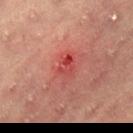Q: Lesion location?
A: the lower back
Q: Illumination type?
A: cross-polarized
Q: How was this image acquired?
A: ~15 mm crop, total-body skin-cancer survey
Q: What are the patient's age and sex?
A: female, aged around 60
Q: Lesion size?
A: about 2.5 mm